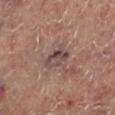This lesion was catalogued during total-body skin photography and was not selected for biopsy.
A close-up tile cropped from a whole-body skin photograph, about 15 mm across.
On the left lower leg.
This is a cross-polarized tile.
Approximately 3.5 mm at its widest.